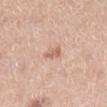biopsy status = no biopsy performed (imaged during a skin exam); image = 15 mm crop, total-body photography; lesion diameter = ≈2.5 mm; subject = female, aged 53 to 57; anatomic site = the right lower leg.